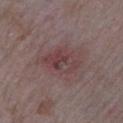{
  "biopsy_status": "not biopsied; imaged during a skin examination",
  "automated_metrics": {
    "nevus_likeness_0_100": 0,
    "lesion_detection_confidence_0_100": 100
  },
  "patient": {
    "sex": "male",
    "age_approx": 65
  },
  "site": "left upper arm",
  "lighting": "white-light",
  "image": {
    "source": "total-body photography crop",
    "field_of_view_mm": 15
  }
}